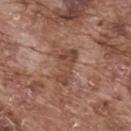follow-up: total-body-photography surveillance lesion; no biopsy.